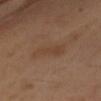workup: catalogued during a skin exam; not biopsied | tile lighting: cross-polarized | location: the arm | patient: female, about 40 years old | lesion diameter: ~4.5 mm (longest diameter) | automated metrics: a border-irregularity rating of about 4/10 and internal color variation of about 1.5 on a 0–10 scale | imaging modality: total-body-photography crop, ~15 mm field of view.